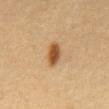Q: Is there a histopathology result?
A: imaged on a skin check; not biopsied
Q: What is the imaging modality?
A: ~15 mm crop, total-body skin-cancer survey
Q: What is the anatomic site?
A: the abdomen
Q: What did automated image analysis measure?
A: an average lesion color of about L≈44 a*≈18 b*≈34 (CIELAB) and about 12 CIELAB-L* units darker than the surrounding skin; an automated nevus-likeness rating near 100 out of 100 and a lesion-detection confidence of about 100/100
Q: What are the patient's age and sex?
A: female, about 50 years old
Q: Illumination type?
A: cross-polarized illumination